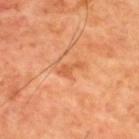Q: Is there a histopathology result?
A: total-body-photography surveillance lesion; no biopsy
Q: What did automated image analysis measure?
A: a normalized lesion–skin contrast near 5.5; a color-variation rating of about 0.5/10; an automated nevus-likeness rating near 0 out of 100 and a detector confidence of about 100 out of 100 that the crop contains a lesion
Q: Where on the body is the lesion?
A: the upper back
Q: What is the imaging modality?
A: ~15 mm crop, total-body skin-cancer survey
Q: Patient demographics?
A: male, aged around 60
Q: How was the tile lit?
A: cross-polarized illumination
Q: How large is the lesion?
A: ≈3 mm